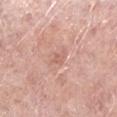follow-up = total-body-photography surveillance lesion; no biopsy | subject = female, aged 63–67 | location = the left lower leg | lighting = white-light illumination | size = about 2.5 mm | acquisition = ~15 mm crop, total-body skin-cancer survey.